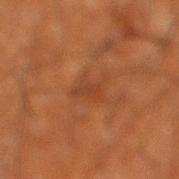Background: On the right lower leg. The patient is a male aged 63–67. This image is a 15 mm lesion crop taken from a total-body photograph. Approximately 4 mm at its widest. Captured under cross-polarized illumination.